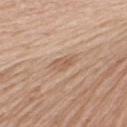| key | value |
|---|---|
| workup | imaged on a skin check; not biopsied |
| patient | female, aged around 75 |
| lesion diameter | about 2.5 mm |
| acquisition | ~15 mm crop, total-body skin-cancer survey |
| tile lighting | white-light |
| location | the upper back |
| automated lesion analysis | a border-irregularity rating of about 3/10, internal color variation of about 3 on a 0–10 scale, and a peripheral color-asymmetry measure near 1; an automated nevus-likeness rating near 0 out of 100 and a detector confidence of about 100 out of 100 that the crop contains a lesion |